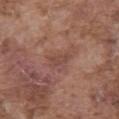Notes:
- notes · total-body-photography surveillance lesion; no biopsy
- anatomic site · the abdomen
- acquisition · ~15 mm tile from a whole-body skin photo
- patient · male, in their mid-70s
- lesion diameter · ≈3 mm
- tile lighting · white-light illumination